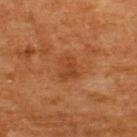An algorithmic analysis of the crop reported a lesion area of about 5.5 mm², an outline eccentricity of about 0.55 (0 = round, 1 = elongated), and a shape-asymmetry score of about 0.3 (0 = symmetric). And it measured a lesion color around L≈40 a*≈26 b*≈37 in CIELAB, roughly 6 lightness units darker than nearby skin, and a normalized border contrast of about 5.5. Longest diameter approximately 3 mm. A 15 mm crop from a total-body photograph taken for skin-cancer surveillance. The lesion is located on the back. Imaged with cross-polarized lighting. The patient is a male aged approximately 60.